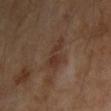biopsy status = no biopsy performed (imaged during a skin exam); lesion size = about 3.5 mm; patient = in their mid- to late 50s; illumination = cross-polarized illumination; site = the right upper arm; image = ~15 mm crop, total-body skin-cancer survey.